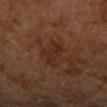A close-up tile cropped from a whole-body skin photograph, about 15 mm across.
The lesion's longest dimension is about 4 mm.
Captured under cross-polarized illumination.
The subject is a female about 60 years old.
The lesion is on the right upper arm.